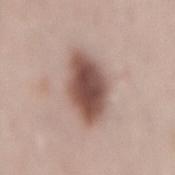– biopsy status · imaged on a skin check; not biopsied
– image · total-body-photography crop, ~15 mm field of view
– subject · female, approximately 30 years of age
– size · ≈6.5 mm
– tile lighting · white-light illumination
– site · the mid back